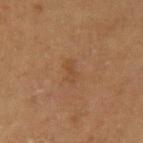This lesion was catalogued during total-body skin photography and was not selected for biopsy. Cropped from a whole-body photographic skin survey; the tile spans about 15 mm. On the left upper arm. This is a cross-polarized tile. An algorithmic analysis of the crop reported a footprint of about 2.5 mm², an outline eccentricity of about 0.9 (0 = round, 1 = elongated), and a symmetry-axis asymmetry near 0.3. The analysis additionally found border irregularity of about 3.5 on a 0–10 scale, internal color variation of about 0 on a 0–10 scale, and a peripheral color-asymmetry measure near 0. And it measured a nevus-likeness score of about 0/100 and lesion-presence confidence of about 100/100. The patient is a male approximately 60 years of age.